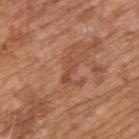Clinical impression: The lesion was tiled from a total-body skin photograph and was not biopsied. Background: On the upper back. An algorithmic analysis of the crop reported an area of roughly 3.5 mm², an eccentricity of roughly 0.95, and a shape-asymmetry score of about 0.45 (0 = symmetric). The analysis additionally found border irregularity of about 5.5 on a 0–10 scale, internal color variation of about 0 on a 0–10 scale, and a peripheral color-asymmetry measure near 0. The recorded lesion diameter is about 3.5 mm. A male patient, about 65 years old. A 15 mm crop from a total-body photograph taken for skin-cancer surveillance. This is a white-light tile.